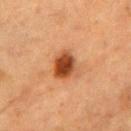Assessment:
Imaged during a routine full-body skin examination; the lesion was not biopsied and no histopathology is available.
Image and clinical context:
A region of skin cropped from a whole-body photographic capture, roughly 15 mm wide. A male patient roughly 85 years of age or older. Approximately 3.5 mm at its widest. On the front of the torso.